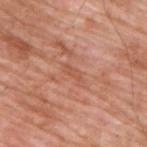follow-up: total-body-photography surveillance lesion; no biopsy | subject: male, about 60 years old | lighting: white-light illumination | image source: ~15 mm crop, total-body skin-cancer survey | lesion size: ~3 mm (longest diameter) | location: the upper back.